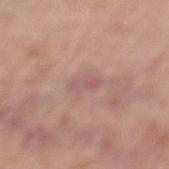Impression:
Part of a total-body skin-imaging series; this lesion was reviewed on a skin check and was not flagged for biopsy.
Image and clinical context:
A female patient approximately 70 years of age. A 15 mm close-up tile from a total-body photography series done for melanoma screening. The lesion is located on the left lower leg. Automated tile analysis of the lesion measured a footprint of about 4 mm², an outline eccentricity of about 0.85 (0 = round, 1 = elongated), and two-axis asymmetry of about 0.3. It also reported a mean CIELAB color near L≈57 a*≈21 b*≈21, a lesion–skin lightness drop of about 6, and a normalized border contrast of about 5. And it measured a border-irregularity rating of about 3/10, internal color variation of about 4.5 on a 0–10 scale, and peripheral color asymmetry of about 1.5. And it measured a nevus-likeness score of about 0/100 and a lesion-detection confidence of about 80/100. The lesion's longest dimension is about 3 mm.